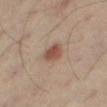{"biopsy_status": "not biopsied; imaged during a skin examination", "lesion_size": {"long_diameter_mm_approx": 3.0}, "patient": {"sex": "male", "age_approx": 50}, "image": {"source": "total-body photography crop", "field_of_view_mm": 15}, "site": "right thigh", "automated_metrics": {"area_mm2_approx": 5.5, "eccentricity": 0.6, "shape_asymmetry": 0.2, "vs_skin_contrast_norm": 8.0}, "lighting": "cross-polarized"}